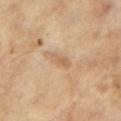<lesion>
  <image>
    <source>total-body photography crop</source>
    <field_of_view_mm>15</field_of_view_mm>
  </image>
  <patient>
    <sex>male</sex>
    <age_approx>65</age_approx>
  </patient>
</lesion>